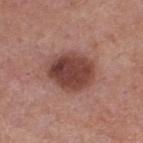notes — imaged on a skin check; not biopsied
lighting — white-light illumination
location — the upper back
subject — female, aged approximately 60
lesion diameter — ≈5.5 mm
acquisition — ~15 mm tile from a whole-body skin photo